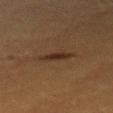Q: Was a biopsy performed?
A: catalogued during a skin exam; not biopsied
Q: What kind of image is this?
A: ~15 mm tile from a whole-body skin photo
Q: What lighting was used for the tile?
A: cross-polarized
Q: Lesion size?
A: ~3 mm (longest diameter)
Q: Patient demographics?
A: female, about 55 years old
Q: Lesion location?
A: the mid back